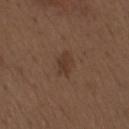Impression:
Part of a total-body skin-imaging series; this lesion was reviewed on a skin check and was not flagged for biopsy.
Background:
A 15 mm crop from a total-body photograph taken for skin-cancer surveillance. A male subject, aged around 70. From the right upper arm.